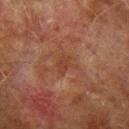biopsy status: no biopsy performed (imaged during a skin exam)
image-analysis metrics: a shape eccentricity near 0.7 and a symmetry-axis asymmetry near 0.3; a lesion color around L≈33 a*≈20 b*≈27 in CIELAB
image: ~15 mm crop, total-body skin-cancer survey
location: the left forearm
illumination: cross-polarized illumination
subject: male, roughly 75 years of age
lesion diameter: about 2.5 mm